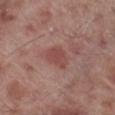Impression:
Captured during whole-body skin photography for melanoma surveillance; the lesion was not biopsied.
Image and clinical context:
From the right lower leg. Approximately 3.5 mm at its widest. A region of skin cropped from a whole-body photographic capture, roughly 15 mm wide. The subject is a male about 70 years old.